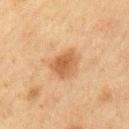Findings:
- follow-up — no biopsy performed (imaged during a skin exam)
- image source — total-body-photography crop, ~15 mm field of view
- location — the right upper arm
- subject — male, aged approximately 65
- tile lighting — cross-polarized